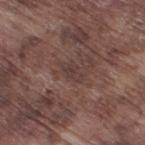<lesion>
<biopsy_status>not biopsied; imaged during a skin examination</biopsy_status>
<lesion_size>
  <long_diameter_mm_approx>3.0</long_diameter_mm_approx>
</lesion_size>
<patient>
  <sex>male</sex>
  <age_approx>75</age_approx>
</patient>
<image>
  <source>total-body photography crop</source>
  <field_of_view_mm>15</field_of_view_mm>
</image>
<lighting>white-light</lighting>
<automated_metrics>
  <area_mm2_approx>3.5</area_mm2_approx>
  <shape_asymmetry>0.4</shape_asymmetry>
  <nevus_likeness_0_100>0</nevus_likeness_0_100>
  <lesion_detection_confidence_0_100>90</lesion_detection_confidence_0_100>
</automated_metrics>
<site>right thigh</site>
</lesion>